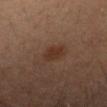This lesion was catalogued during total-body skin photography and was not selected for biopsy. This image is a 15 mm lesion crop taken from a total-body photograph. From the head or neck. A male patient, aged around 30.